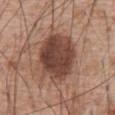• follow-up · imaged on a skin check; not biopsied